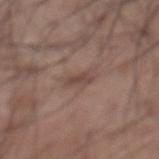follow-up: imaged on a skin check; not biopsied
body site: the abdomen
subject: male, aged 53–57
illumination: white-light illumination
lesion diameter: ≈2.5 mm
imaging modality: 15 mm crop, total-body photography